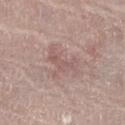follow-up = no biopsy performed (imaged during a skin exam) | location = the right thigh | subject = female, aged 63 to 67 | image = 15 mm crop, total-body photography.